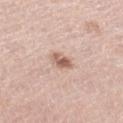Q: What is the anatomic site?
A: the left thigh
Q: Automated lesion metrics?
A: a footprint of about 4 mm²; a mean CIELAB color near L≈60 a*≈20 b*≈27 and a normalized lesion–skin contrast near 8.5; a classifier nevus-likeness of about 70/100 and a detector confidence of about 100 out of 100 that the crop contains a lesion
Q: What kind of image is this?
A: ~15 mm crop, total-body skin-cancer survey
Q: What is the lesion's diameter?
A: ≈2.5 mm
Q: Patient demographics?
A: female, in their mid- to late 60s
Q: What lighting was used for the tile?
A: white-light illumination